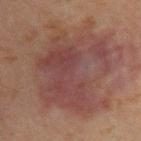{"lesion_size": {"long_diameter_mm_approx": 9.5}, "patient": {"sex": "male", "age_approx": 30}, "image": {"source": "total-body photography crop", "field_of_view_mm": 15}, "site": "upper back", "lighting": "cross-polarized"}